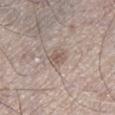Automated image analysis of the tile measured an average lesion color of about L≈56 a*≈14 b*≈23 (CIELAB), a lesion–skin lightness drop of about 9, and a lesion-to-skin contrast of about 6 (normalized; higher = more distinct). The software also gave border irregularity of about 2.5 on a 0–10 scale, a within-lesion color-variation index near 2/10, and radial color variation of about 0.5. The subject is a male about 70 years old. The tile uses white-light illumination. Approximately 2.5 mm at its widest. The lesion is located on the left lower leg. A lesion tile, about 15 mm wide, cut from a 3D total-body photograph.